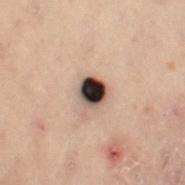No biopsy was performed on this lesion — it was imaged during a full skin examination and was not determined to be concerning.
The patient is a female in their mid-50s.
The lesion is on the left leg.
Automated tile analysis of the lesion measured an area of roughly 6 mm², an eccentricity of roughly 0.3, and a symmetry-axis asymmetry near 0.1. The software also gave a lesion–skin lightness drop of about 26. The analysis additionally found an automated nevus-likeness rating near 35 out of 100 and a lesion-detection confidence of about 100/100.
A region of skin cropped from a whole-body photographic capture, roughly 15 mm wide.
This is a cross-polarized tile.